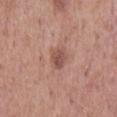Impression: No biopsy was performed on this lesion — it was imaged during a full skin examination and was not determined to be concerning. Clinical summary: A male patient in their 60s. The tile uses white-light illumination. Automated tile analysis of the lesion measured an area of roughly 4.5 mm², an outline eccentricity of about 0.8 (0 = round, 1 = elongated), and a symmetry-axis asymmetry near 0.2. It also reported a mean CIELAB color near L≈51 a*≈22 b*≈25, a lesion–skin lightness drop of about 10, and a normalized border contrast of about 7.5. The software also gave border irregularity of about 2 on a 0–10 scale and a within-lesion color-variation index near 3.5/10. And it measured a lesion-detection confidence of about 100/100. A region of skin cropped from a whole-body photographic capture, roughly 15 mm wide. Located on the mid back.